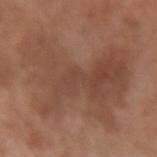Clinical impression:
Part of a total-body skin-imaging series; this lesion was reviewed on a skin check and was not flagged for biopsy.
Background:
The lesion is located on the right forearm. A female subject, about 50 years old. Cropped from a total-body skin-imaging series; the visible field is about 15 mm. Measured at roughly 12 mm in maximum diameter. The tile uses white-light illumination.